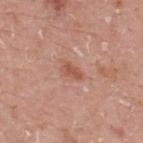No biopsy was performed on this lesion — it was imaged during a full skin examination and was not determined to be concerning. The lesion-visualizer software estimated a lesion area of about 3.5 mm² and two-axis asymmetry of about 0.25. Located on the upper back. A 15 mm close-up extracted from a 3D total-body photography capture. Measured at roughly 2.5 mm in maximum diameter. A male subject, roughly 75 years of age.